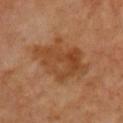Captured during whole-body skin photography for melanoma surveillance; the lesion was not biopsied. A male subject, aged approximately 65. The tile uses cross-polarized illumination. The lesion-visualizer software estimated border irregularity of about 4 on a 0–10 scale and internal color variation of about 4.5 on a 0–10 scale. The analysis additionally found a nevus-likeness score of about 0/100. Longest diameter approximately 6.5 mm. A 15 mm close-up extracted from a 3D total-body photography capture. From the chest.